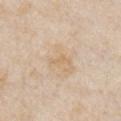Q: Is there a histopathology result?
A: imaged on a skin check; not biopsied
Q: What is the lesion's diameter?
A: ~2.5 mm (longest diameter)
Q: What are the patient's age and sex?
A: male, aged around 75
Q: Where on the body is the lesion?
A: the arm
Q: How was this image acquired?
A: 15 mm crop, total-body photography
Q: How was the tile lit?
A: white-light
Q: Automated lesion metrics?
A: a footprint of about 2.5 mm², an eccentricity of roughly 0.9, and a shape-asymmetry score of about 0.35 (0 = symmetric); a border-irregularity index near 4/10, internal color variation of about 0 on a 0–10 scale, and radial color variation of about 0